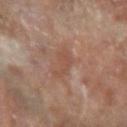Notes:
* follow-up — no biopsy performed (imaged during a skin exam)
* TBP lesion metrics — a footprint of about 5 mm², an outline eccentricity of about 0.9 (0 = round, 1 = elongated), and a shape-asymmetry score of about 0.5 (0 = symmetric); an average lesion color of about L≈47 a*≈19 b*≈27 (CIELAB), roughly 6 lightness units darker than nearby skin, and a lesion-to-skin contrast of about 5 (normalized; higher = more distinct); a border-irregularity index near 5/10, a within-lesion color-variation index near 1.5/10, and radial color variation of about 0.5; a classifier nevus-likeness of about 0/100 and a detector confidence of about 100 out of 100 that the crop contains a lesion
* body site — the arm
* subject — male, approximately 85 years of age or older
* image — ~15 mm crop, total-body skin-cancer survey
* diameter — ≈4 mm
* lighting — cross-polarized